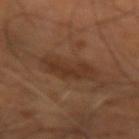Part of a total-body skin-imaging series; this lesion was reviewed on a skin check and was not flagged for biopsy.
Measured at roughly 5 mm in maximum diameter.
A patient in their mid- to late 60s.
A roughly 15 mm field-of-view crop from a total-body skin photograph.
The lesion is located on the left forearm.